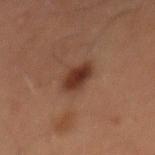biopsy status: catalogued during a skin exam; not biopsied
body site: the mid back
acquisition: ~15 mm crop, total-body skin-cancer survey
patient: male, aged 58 to 62